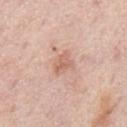Clinical impression:
The lesion was photographed on a routine skin check and not biopsied; there is no pathology result.
Context:
A male patient aged around 75. This image is a 15 mm lesion crop taken from a total-body photograph. Automated image analysis of the tile measured an average lesion color of about L≈63 a*≈22 b*≈29 (CIELAB), roughly 9 lightness units darker than nearby skin, and a lesion-to-skin contrast of about 6 (normalized; higher = more distinct). The software also gave a border-irregularity rating of about 3/10 and a within-lesion color-variation index near 3/10. The software also gave a detector confidence of about 100 out of 100 that the crop contains a lesion. The tile uses white-light illumination. The lesion's longest dimension is about 2.5 mm. On the back.